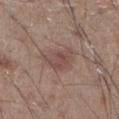Impression: Captured during whole-body skin photography for melanoma surveillance; the lesion was not biopsied. Image and clinical context: The lesion's longest dimension is about 4 mm. Captured under white-light illumination. Cropped from a total-body skin-imaging series; the visible field is about 15 mm. On the right lower leg. The subject is a male aged approximately 60.